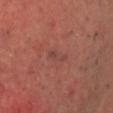<lesion>
<biopsy_status>not biopsied; imaged during a skin examination</biopsy_status>
</lesion>